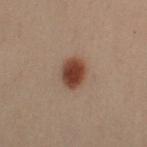Assessment:
No biopsy was performed on this lesion — it was imaged during a full skin examination and was not determined to be concerning.
Image and clinical context:
Imaged with cross-polarized lighting. The lesion is on the left forearm. About 3.5 mm across. A male patient, in their 50s. Cropped from a total-body skin-imaging series; the visible field is about 15 mm. The total-body-photography lesion software estimated an area of roughly 8 mm². And it measured an average lesion color of about L≈33 a*≈18 b*≈23 (CIELAB) and about 12 CIELAB-L* units darker than the surrounding skin. And it measured border irregularity of about 1.5 on a 0–10 scale, a color-variation rating of about 4/10, and a peripheral color-asymmetry measure near 1. And it measured a lesion-detection confidence of about 100/100.